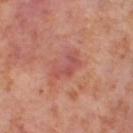| key | value |
|---|---|
| workup | no biopsy performed (imaged during a skin exam) |
| automated metrics | an average lesion color of about L≈53 a*≈29 b*≈26 (CIELAB), roughly 7 lightness units darker than nearby skin, and a lesion-to-skin contrast of about 5.5 (normalized; higher = more distinct) |
| lighting | cross-polarized |
| site | the right thigh |
| subject | female, aged 53 to 57 |
| diameter | ≈4.5 mm |
| image source | 15 mm crop, total-body photography |